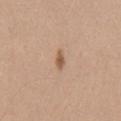notes = catalogued during a skin exam; not biopsied | image source = ~15 mm tile from a whole-body skin photo | patient = male, in their mid-20s | diameter = about 2.5 mm | anatomic site = the chest | automated metrics = a footprint of about 2.5 mm² and an eccentricity of roughly 0.9; a lesion–skin lightness drop of about 11; a border-irregularity rating of about 4/10, a color-variation rating of about 0/10, and peripheral color asymmetry of about 0 | illumination = white-light illumination.